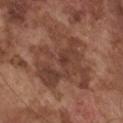The lesion was photographed on a routine skin check and not biopsied; there is no pathology result.
A roughly 15 mm field-of-view crop from a total-body skin photograph.
A male subject aged 73 to 77.
On the front of the torso.